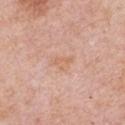A region of skin cropped from a whole-body photographic capture, roughly 15 mm wide.
A male patient, aged 73–77.
Located on the arm.